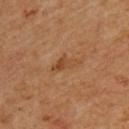biopsy status: imaged on a skin check; not biopsied | patient: male, aged approximately 60 | body site: the upper back | acquisition: ~15 mm tile from a whole-body skin photo | TBP lesion metrics: a nevus-likeness score of about 0/100 and a detector confidence of about 100 out of 100 that the crop contains a lesion | illumination: cross-polarized illumination.